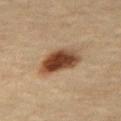Recorded during total-body skin imaging; not selected for excision or biopsy. A 15 mm close-up tile from a total-body photography series done for melanoma screening. Located on the right thigh. The patient is a female aged 63 to 67.